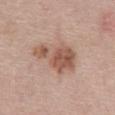Context:
Automated image analysis of the tile measured internal color variation of about 4 on a 0–10 scale and a peripheral color-asymmetry measure near 1.5. A male patient, aged 58 to 62. This is a white-light tile. Cropped from a total-body skin-imaging series; the visible field is about 15 mm. The lesion's longest dimension is about 6 mm. From the abdomen.